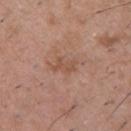The lesion was photographed on a routine skin check and not biopsied; there is no pathology result. A 15 mm close-up extracted from a 3D total-body photography capture. From the upper back. The patient is a male approximately 50 years of age.